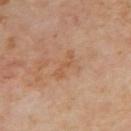Imaged during a routine full-body skin examination; the lesion was not biopsied and no histopathology is available. A female subject, aged 58 to 62. Approximately 3.5 mm at its widest. The lesion is on the upper back. This is a cross-polarized tile. The total-body-photography lesion software estimated a lesion area of about 3.5 mm². It also reported a border-irregularity index near 6/10, internal color variation of about 0 on a 0–10 scale, and peripheral color asymmetry of about 0. A roughly 15 mm field-of-view crop from a total-body skin photograph.